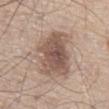Image and clinical context: From the abdomen. A male subject, aged 78 to 82. A 15 mm close-up extracted from a 3D total-body photography capture.